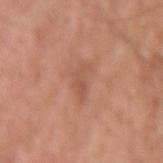subject: male, aged around 55
illumination: white-light illumination
automated metrics: a border-irregularity index near 5.5/10 and a within-lesion color-variation index near 1.5/10; a detector confidence of about 100 out of 100 that the crop contains a lesion
acquisition: 15 mm crop, total-body photography
anatomic site: the left upper arm
lesion diameter: ≈3.5 mm